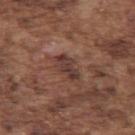Part of a total-body skin-imaging series; this lesion was reviewed on a skin check and was not flagged for biopsy. A region of skin cropped from a whole-body photographic capture, roughly 15 mm wide. A male patient aged 73 to 77. The lesion is on the left upper arm.